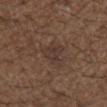follow-up — no biopsy performed (imaged during a skin exam)
TBP lesion metrics — a lesion area of about 6.5 mm², an outline eccentricity of about 0.65 (0 = round, 1 = elongated), and two-axis asymmetry of about 0.45; a color-variation rating of about 2/10 and peripheral color asymmetry of about 0.5; a classifier nevus-likeness of about 0/100
lesion size — ≈3.5 mm
patient — male, about 50 years old
body site — the chest
tile lighting — white-light
image source — 15 mm crop, total-body photography